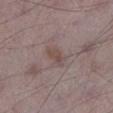automated metrics: an area of roughly 4 mm² and a symmetry-axis asymmetry near 0.35; an average lesion color of about L≈47 a*≈15 b*≈20 (CIELAB) and a lesion–skin lightness drop of about 7; a border-irregularity index near 3.5/10, a within-lesion color-variation index near 2.5/10, and peripheral color asymmetry of about 1 | lighting: white-light | subject: male, aged around 70 | size: ~3 mm (longest diameter) | location: the left lower leg | acquisition: total-body-photography crop, ~15 mm field of view.